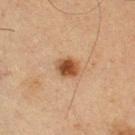{"biopsy_status": "not biopsied; imaged during a skin examination", "lesion_size": {"long_diameter_mm_approx": 3.0}, "site": "right thigh", "patient": {"sex": "male", "age_approx": 65}, "image": {"source": "total-body photography crop", "field_of_view_mm": 15}}